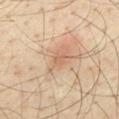follow-up=no biopsy performed (imaged during a skin exam) | site=the mid back | diameter=~1.5 mm (longest diameter) | tile lighting=cross-polarized | image=total-body-photography crop, ~15 mm field of view | patient=male, aged approximately 40.